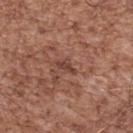| feature | finding |
|---|---|
| notes | imaged on a skin check; not biopsied |
| patient | male, in their mid-50s |
| anatomic site | the upper back |
| acquisition | total-body-photography crop, ~15 mm field of view |